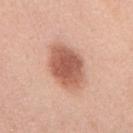Notes:
- biopsy status · total-body-photography surveillance lesion; no biopsy
- image · total-body-photography crop, ~15 mm field of view
- patient · female, approximately 40 years of age
- body site · the upper back